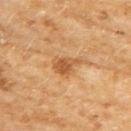  biopsy_status: not biopsied; imaged during a skin examination
  site: upper back
  image:
    source: total-body photography crop
    field_of_view_mm: 15
  patient:
    sex: female
    age_approx: 60